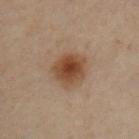Part of a total-body skin-imaging series; this lesion was reviewed on a skin check and was not flagged for biopsy.
Approximately 4 mm at its widest.
Automated tile analysis of the lesion measured a border-irregularity index near 2/10, a color-variation rating of about 4.5/10, and a peripheral color-asymmetry measure near 1.
A male patient, in their mid- to late 50s.
On the right upper arm.
A region of skin cropped from a whole-body photographic capture, roughly 15 mm wide.